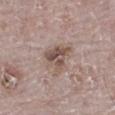Notes:
– workup · catalogued during a skin exam; not biopsied
– patient · male, about 70 years old
– diameter · about 4 mm
– image source · ~15 mm crop, total-body skin-cancer survey
– location · the right leg